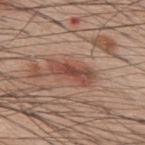No biopsy was performed on this lesion — it was imaged during a full skin examination and was not determined to be concerning. A male patient aged around 60. This image is a 15 mm lesion crop taken from a total-body photograph. Captured under white-light illumination. Approximately 5 mm at its widest. The lesion is on the upper back.